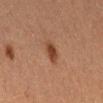This image is a 15 mm lesion crop taken from a total-body photograph.
From the right thigh.
A female subject in their 40s.